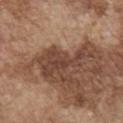follow-up: no biopsy performed (imaged during a skin exam)
subject: male, roughly 75 years of age
size: ~7 mm (longest diameter)
body site: the chest
acquisition: total-body-photography crop, ~15 mm field of view